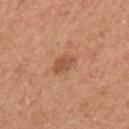This lesion was catalogued during total-body skin photography and was not selected for biopsy. A female patient, in their 40s. Longest diameter approximately 2.5 mm. On the left upper arm. Imaged with white-light lighting. A 15 mm close-up tile from a total-body photography series done for melanoma screening.